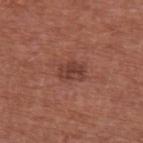Assessment: This lesion was catalogued during total-body skin photography and was not selected for biopsy. Acquisition and patient details: A 15 mm crop from a total-body photograph taken for skin-cancer surveillance. On the left upper arm. The patient is a male about 65 years old.